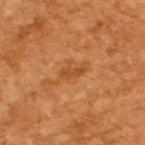This image is a 15 mm lesion crop taken from a total-body photograph.
A male subject aged approximately 65.